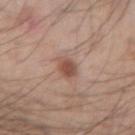The lesion was photographed on a routine skin check and not biopsied; there is no pathology result.
A 15 mm close-up tile from a total-body photography series done for melanoma screening.
Located on the left forearm.
A male patient aged approximately 35.